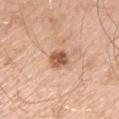Imaged during a routine full-body skin examination; the lesion was not biopsied and no histopathology is available.
Measured at roughly 2.5 mm in maximum diameter.
Captured under white-light illumination.
On the left upper arm.
This image is a 15 mm lesion crop taken from a total-body photograph.
A male patient in their mid-50s.